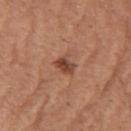| key | value |
|---|---|
| notes | total-body-photography surveillance lesion; no biopsy |
| illumination | white-light illumination |
| lesion size | ≈2.5 mm |
| TBP lesion metrics | a border-irregularity index near 2.5/10, a color-variation rating of about 3.5/10, and peripheral color asymmetry of about 1.5 |
| site | the chest |
| patient | female, aged around 65 |
| imaging modality | ~15 mm crop, total-body skin-cancer survey |